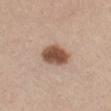Located on the mid back.
Approximately 4 mm at its widest.
The total-body-photography lesion software estimated a shape eccentricity near 0.75 and two-axis asymmetry of about 0.2. The software also gave border irregularity of about 2 on a 0–10 scale. And it measured a nevus-likeness score of about 100/100 and lesion-presence confidence of about 100/100.
The subject is a female roughly 40 years of age.
This image is a 15 mm lesion crop taken from a total-body photograph.